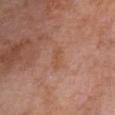Case summary:
- biopsy status · total-body-photography surveillance lesion; no biopsy
- lesion diameter · ≈2.5 mm
- body site · the chest
- image source · total-body-photography crop, ~15 mm field of view
- automated lesion analysis · a mean CIELAB color near L≈52 a*≈23 b*≈33, roughly 6 lightness units darker than nearby skin, and a normalized lesion–skin contrast near 5.5
- subject · male, aged 58–62
- illumination · white-light illumination